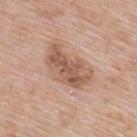Captured during whole-body skin photography for melanoma surveillance; the lesion was not biopsied.
On the back.
Longest diameter approximately 6 mm.
A 15 mm close-up tile from a total-body photography series done for melanoma screening.
A male patient approximately 65 years of age.
The tile uses white-light illumination.